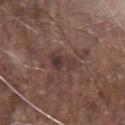biopsy status: no biopsy performed (imaged during a skin exam) | subject: male, approximately 80 years of age | lighting: white-light illumination | image source: ~15 mm crop, total-body skin-cancer survey | anatomic site: the chest | size: ≈3.5 mm.